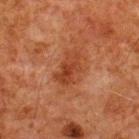Impression: The lesion was photographed on a routine skin check and not biopsied; there is no pathology result. Acquisition and patient details: The patient is a male aged 78 to 82. A 15 mm close-up tile from a total-body photography series done for melanoma screening. From the back. The tile uses cross-polarized illumination.